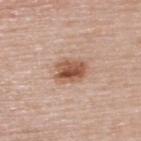Assessment:
This lesion was catalogued during total-body skin photography and was not selected for biopsy.
Image and clinical context:
The lesion is on the upper back. The lesion's longest dimension is about 3.5 mm. A female patient about 50 years old. A close-up tile cropped from a whole-body skin photograph, about 15 mm across. The lesion-visualizer software estimated a lesion color around L≈54 a*≈21 b*≈31 in CIELAB and about 14 CIELAB-L* units darker than the surrounding skin. It also reported a border-irregularity rating of about 2/10, a within-lesion color-variation index near 7/10, and peripheral color asymmetry of about 2.5. Captured under white-light illumination.